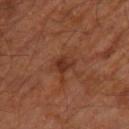Part of a total-body skin-imaging series; this lesion was reviewed on a skin check and was not flagged for biopsy. The patient is a male aged 58 to 62. From the right leg. The recorded lesion diameter is about 2.5 mm. This image is a 15 mm lesion crop taken from a total-body photograph. The total-body-photography lesion software estimated a border-irregularity index near 2/10 and internal color variation of about 3 on a 0–10 scale.